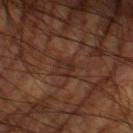| feature | finding |
|---|---|
| anatomic site | the left thigh |
| image source | ~15 mm crop, total-body skin-cancer survey |
| subject | male, aged 58–62 |
| tile lighting | cross-polarized |